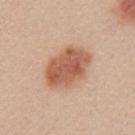The lesion was tiled from a total-body skin photograph and was not biopsied. This is a white-light tile. This image is a 15 mm lesion crop taken from a total-body photograph. A female subject approximately 25 years of age. The lesion is located on the left upper arm.